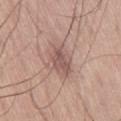Captured during whole-body skin photography for melanoma surveillance; the lesion was not biopsied. The patient is a male aged around 60. About 4.5 mm across. Cropped from a total-body skin-imaging series; the visible field is about 15 mm. The tile uses white-light illumination. From the leg.